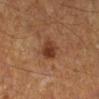The lesion was photographed on a routine skin check and not biopsied; there is no pathology result. A male patient aged 58–62. A roughly 15 mm field-of-view crop from a total-body skin photograph. The total-body-photography lesion software estimated a footprint of about 5.5 mm², an eccentricity of roughly 0.45, and a shape-asymmetry score of about 0.2 (0 = symmetric). The analysis additionally found a lesion–skin lightness drop of about 10. And it measured a classifier nevus-likeness of about 90/100 and a lesion-detection confidence of about 100/100. On the right lower leg. This is a cross-polarized tile. About 3 mm across.